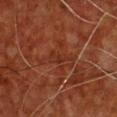Recorded during total-body skin imaging; not selected for excision or biopsy. A male subject roughly 60 years of age. On the chest. Cropped from a total-body skin-imaging series; the visible field is about 15 mm.